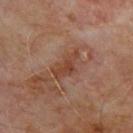notes = catalogued during a skin exam; not biopsied | illumination = cross-polarized | image = ~15 mm crop, total-body skin-cancer survey | TBP lesion metrics = an average lesion color of about L≈41 a*≈21 b*≈28 (CIELAB), roughly 8 lightness units darker than nearby skin, and a normalized border contrast of about 7; a border-irregularity index near 5/10, internal color variation of about 2.5 on a 0–10 scale, and radial color variation of about 0.5 | body site = the upper back | patient = male, in their mid-60s | lesion diameter = ~4 mm (longest diameter).